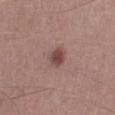Notes:
- lesion size · ≈2.5 mm
- image-analysis metrics · a shape-asymmetry score of about 0.2 (0 = symmetric)
- site · the left lower leg
- patient · male, aged approximately 45
- image · 15 mm crop, total-body photography
- lighting · white-light